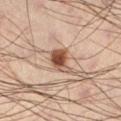| feature | finding |
|---|---|
| biopsy status | catalogued during a skin exam; not biopsied |
| subject | male, roughly 40 years of age |
| image source | ~15 mm tile from a whole-body skin photo |
| site | the leg |